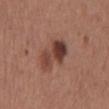<tbp_lesion>
<biopsy_status>not biopsied; imaged during a skin examination</biopsy_status>
<image>
  <source>total-body photography crop</source>
  <field_of_view_mm>15</field_of_view_mm>
</image>
<site>mid back</site>
<lesion_size>
  <long_diameter_mm_approx>5.5</long_diameter_mm_approx>
</lesion_size>
<patient>
  <sex>male</sex>
  <age_approx>65</age_approx>
</patient>
</tbp_lesion>